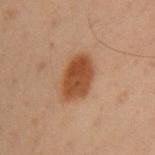Captured during whole-body skin photography for melanoma surveillance; the lesion was not biopsied.
The total-body-photography lesion software estimated an outline eccentricity of about 0.7 (0 = round, 1 = elongated) and two-axis asymmetry of about 0.15. The software also gave a border-irregularity index near 1.5/10, a within-lesion color-variation index near 3/10, and peripheral color asymmetry of about 1. And it measured lesion-presence confidence of about 100/100.
Cropped from a whole-body photographic skin survey; the tile spans about 15 mm.
The tile uses cross-polarized illumination.
From the arm.
A female patient, aged 38–42.